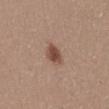notes=no biopsy performed (imaged during a skin exam); body site=the front of the torso; patient=female, about 35 years old; lighting=white-light; lesion diameter=≈3 mm; acquisition=15 mm crop, total-body photography.